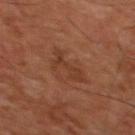Findings:
• workup — catalogued during a skin exam; not biopsied
• diameter — about 3.5 mm
• anatomic site — the chest
• subject — male, aged 58 to 62
• imaging modality — ~15 mm crop, total-body skin-cancer survey
• lighting — cross-polarized